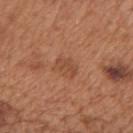  biopsy_status: not biopsied; imaged during a skin examination
  lesion_size:
    long_diameter_mm_approx: 2.5
  patient:
    sex: male
    age_approx: 65
  site: mid back
  image:
    source: total-body photography crop
    field_of_view_mm: 15
  automated_metrics:
    area_mm2_approx: 2.5
    shape_asymmetry: 0.45
    cielab_L: 47
    cielab_a: 24
    cielab_b: 33
    vs_skin_darker_L: 7.0
    vs_skin_contrast_norm: 5.5
    border_irregularity_0_10: 5.0
    peripheral_color_asymmetry: 0.0
    nevus_likeness_0_100: 15
    lesion_detection_confidence_0_100: 100
  lighting: white-light